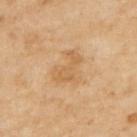Q: Patient demographics?
A: female, about 60 years old
Q: What is the imaging modality?
A: ~15 mm crop, total-body skin-cancer survey
Q: How was the tile lit?
A: cross-polarized illumination
Q: Where on the body is the lesion?
A: the upper back